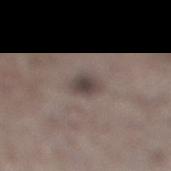Impression:
This lesion was catalogued during total-body skin photography and was not selected for biopsy.
Background:
A 15 mm close-up extracted from a 3D total-body photography capture. The tile uses white-light illumination. Approximately 3 mm at its widest. The lesion is on the right lower leg. A male subject about 70 years old.